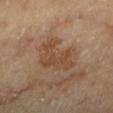anatomic site = the leg
size = ≈6 mm
acquisition = ~15 mm tile from a whole-body skin photo
subject = female, aged 58 to 62